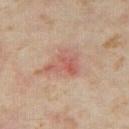Findings:
- follow-up · imaged on a skin check; not biopsied
- lesion size · about 4 mm
- tile lighting · cross-polarized illumination
- acquisition · total-body-photography crop, ~15 mm field of view
- subject · female, aged 33 to 37
- location · the right thigh